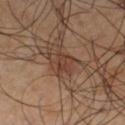Imaged during a routine full-body skin examination; the lesion was not biopsied and no histopathology is available.
A 15 mm close-up extracted from a 3D total-body photography capture.
On the leg.
A male patient, aged around 60.
The tile uses cross-polarized illumination.
Automated tile analysis of the lesion measured an outline eccentricity of about 0.85 (0 = round, 1 = elongated) and a shape-asymmetry score of about 0.35 (0 = symmetric). The software also gave an automated nevus-likeness rating near 60 out of 100 and lesion-presence confidence of about 85/100.
Approximately 2.5 mm at its widest.